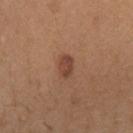Clinical impression:
The lesion was photographed on a routine skin check and not biopsied; there is no pathology result.
Clinical summary:
Cropped from a total-body skin-imaging series; the visible field is about 15 mm. Automated image analysis of the tile measured a lesion color around L≈43 a*≈22 b*≈29 in CIELAB, roughly 10 lightness units darker than nearby skin, and a normalized lesion–skin contrast near 8. The analysis additionally found a classifier nevus-likeness of about 90/100 and a detector confidence of about 100 out of 100 that the crop contains a lesion. The patient is a female aged 53–57. Captured under white-light illumination. The recorded lesion diameter is about 2.5 mm. On the chest.